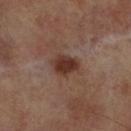Q: Was this lesion biopsied?
A: no biopsy performed (imaged during a skin exam)
Q: What is the lesion's diameter?
A: ~3.5 mm (longest diameter)
Q: What is the anatomic site?
A: the right lower leg
Q: Who is the patient?
A: male, roughly 70 years of age
Q: What is the imaging modality?
A: ~15 mm crop, total-body skin-cancer survey